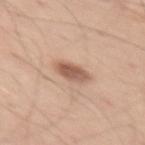biopsy status=imaged on a skin check; not biopsied
lighting=white-light
lesion diameter=≈3.5 mm
location=the left thigh
TBP lesion metrics=a classifier nevus-likeness of about 90/100 and lesion-presence confidence of about 100/100
patient=male, aged around 50
imaging modality=~15 mm crop, total-body skin-cancer survey